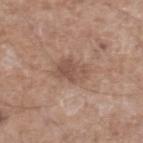Recorded during total-body skin imaging; not selected for excision or biopsy. Measured at roughly 4 mm in maximum diameter. A 15 mm close-up extracted from a 3D total-body photography capture. The lesion is on the right lower leg. This is a white-light tile. A male patient aged 63 to 67.